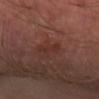{
  "biopsy_status": "not biopsied; imaged during a skin examination",
  "patient": {
    "sex": "male",
    "age_approx": 70
  },
  "automated_metrics": {
    "area_mm2_approx": 3.0,
    "eccentricity": 0.95,
    "color_variation_0_10": 0.0
  },
  "site": "right forearm",
  "lesion_size": {
    "long_diameter_mm_approx": 3.0
  },
  "lighting": "cross-polarized",
  "image": {
    "source": "total-body photography crop",
    "field_of_view_mm": 15
  }
}